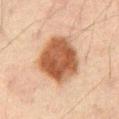This lesion was catalogued during total-body skin photography and was not selected for biopsy.
A 15 mm close-up tile from a total-body photography series done for melanoma screening.
This is a cross-polarized tile.
Automated tile analysis of the lesion measured a lesion area of about 24 mm², an eccentricity of roughly 0.55, and two-axis asymmetry of about 0.15. The analysis additionally found a border-irregularity rating of about 1.5/10, a within-lesion color-variation index near 4.5/10, and a peripheral color-asymmetry measure near 1.5. The software also gave an automated nevus-likeness rating near 100 out of 100 and lesion-presence confidence of about 100/100.
A male subject, in their mid- to late 60s.
From the mid back.